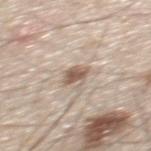{
  "biopsy_status": "not biopsied; imaged during a skin examination",
  "patient": {
    "sex": "male",
    "age_approx": 60
  },
  "lighting": "white-light",
  "lesion_size": {
    "long_diameter_mm_approx": 3.0
  },
  "image": {
    "source": "total-body photography crop",
    "field_of_view_mm": 15
  },
  "automated_metrics": {
    "area_mm2_approx": 4.5,
    "nevus_likeness_0_100": 85,
    "lesion_detection_confidence_0_100": 95
  },
  "site": "back"
}